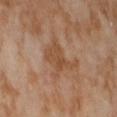| key | value |
|---|---|
| biopsy status | no biopsy performed (imaged during a skin exam) |
| size | about 4.5 mm |
| automated metrics | an area of roughly 10 mm²; a lesion color around L≈50 a*≈20 b*≈33 in CIELAB and a lesion–skin lightness drop of about 7; a classifier nevus-likeness of about 0/100 and a lesion-detection confidence of about 100/100 |
| patient | female, aged 53 to 57 |
| location | the right thigh |
| image | 15 mm crop, total-body photography |